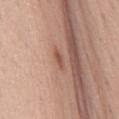  biopsy_status: not biopsied; imaged during a skin examination
  patient:
    sex: female
    age_approx: 65
  site: back
  image:
    source: total-body photography crop
    field_of_view_mm: 15
  automated_metrics:
    area_mm2_approx: 3.0
    eccentricity: 0.9
    shape_asymmetry: 0.3
    cielab_L: 54
    cielab_a: 20
    cielab_b: 29
    vs_skin_darker_L: 10.0
    border_irregularity_0_10: 3.0
    color_variation_0_10: 1.5
    peripheral_color_asymmetry: 0.5
    nevus_likeness_0_100: 5